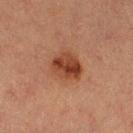The lesion was photographed on a routine skin check and not biopsied; there is no pathology result.
A lesion tile, about 15 mm wide, cut from a 3D total-body photograph.
The subject is a female aged 38 to 42.
The total-body-photography lesion software estimated an average lesion color of about L≈35 a*≈23 b*≈28 (CIELAB) and a normalized border contrast of about 9.5.
On the left thigh.
This is a cross-polarized tile.
Measured at roughly 3.5 mm in maximum diameter.